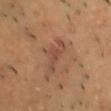workup=no biopsy performed (imaged during a skin exam)
image source=15 mm crop, total-body photography
location=the chest
tile lighting=cross-polarized
patient=male, aged approximately 45